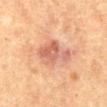| field | value |
|---|---|
| biopsy status | no biopsy performed (imaged during a skin exam) |
| acquisition | total-body-photography crop, ~15 mm field of view |
| automated lesion analysis | a lesion area of about 12 mm² and two-axis asymmetry of about 0.3; a border-irregularity index near 3.5/10, a color-variation rating of about 5.5/10, and radial color variation of about 2; a nevus-likeness score of about 0/100 and a detector confidence of about 100 out of 100 that the crop contains a lesion |
| subject | male, in their mid-60s |
| location | the abdomen |
| lesion size | about 5 mm |
| tile lighting | cross-polarized illumination |